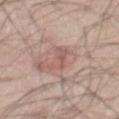Captured during whole-body skin photography for melanoma surveillance; the lesion was not biopsied.
The lesion-visualizer software estimated a lesion color around L≈56 a*≈21 b*≈23 in CIELAB, about 8 CIELAB-L* units darker than the surrounding skin, and a normalized border contrast of about 5.5. And it measured a border-irregularity index near 8/10, a color-variation rating of about 0/10, and a peripheral color-asymmetry measure near 0. It also reported a detector confidence of about 95 out of 100 that the crop contains a lesion.
Captured under white-light illumination.
A male subject approximately 50 years of age.
The lesion is on the mid back.
Approximately 4 mm at its widest.
A 15 mm crop from a total-body photograph taken for skin-cancer surveillance.